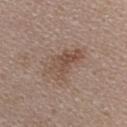workup = imaged on a skin check; not biopsied | anatomic site = the upper back | diameter = ≈5 mm | automated metrics = a nevus-likeness score of about 15/100 and lesion-presence confidence of about 100/100 | lighting = white-light illumination | subject = female, in their mid-40s | imaging modality = ~15 mm tile from a whole-body skin photo.